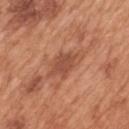The lesion was tiled from a total-body skin photograph and was not biopsied. The tile uses white-light illumination. The total-body-photography lesion software estimated an outline eccentricity of about 0.85 (0 = round, 1 = elongated). And it measured an average lesion color of about L≈51 a*≈26 b*≈32 (CIELAB), about 9 CIELAB-L* units darker than the surrounding skin, and a normalized lesion–skin contrast near 6.5. It also reported a border-irregularity rating of about 3.5/10. Located on the mid back. Cropped from a whole-body photographic skin survey; the tile spans about 15 mm. A male patient in their mid- to late 60s. About 5 mm across.